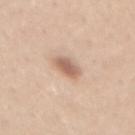Assessment:
Recorded during total-body skin imaging; not selected for excision or biopsy.
Clinical summary:
The lesion is on the mid back. Automated image analysis of the tile measured a footprint of about 5 mm², an outline eccentricity of about 0.7 (0 = round, 1 = elongated), and a symmetry-axis asymmetry near 0.1. And it measured an automated nevus-likeness rating near 90 out of 100. The recorded lesion diameter is about 3 mm. A 15 mm close-up tile from a total-body photography series done for melanoma screening. The subject is a female approximately 45 years of age.